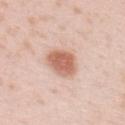follow-up: no biopsy performed (imaged during a skin exam) | lighting: white-light illumination | imaging modality: ~15 mm tile from a whole-body skin photo | lesion diameter: about 4.5 mm | patient: female, roughly 40 years of age | TBP lesion metrics: a nevus-likeness score of about 100/100 and lesion-presence confidence of about 100/100 | anatomic site: the upper back.